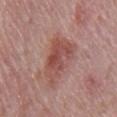<tbp_lesion>
<biopsy_status>not biopsied; imaged during a skin examination</biopsy_status>
<image>
  <source>total-body photography crop</source>
  <field_of_view_mm>15</field_of_view_mm>
</image>
<site>chest</site>
<lesion_size>
  <long_diameter_mm_approx>6.0</long_diameter_mm_approx>
</lesion_size>
<lighting>white-light</lighting>
<patient>
  <sex>male</sex>
  <age_approx>65</age_approx>
</patient>
</tbp_lesion>